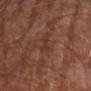notes: total-body-photography surveillance lesion; no biopsy | automated lesion analysis: an outline eccentricity of about 0.95 (0 = round, 1 = elongated) and two-axis asymmetry of about 0.5; a border-irregularity rating of about 5.5/10 and a color-variation rating of about 0/10; lesion-presence confidence of about 55/100 | anatomic site: the right forearm | image: ~15 mm tile from a whole-body skin photo | patient: male, aged approximately 70.